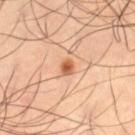Notes:
* location — the left thigh
* patient — male, in their mid- to late 60s
* imaging modality — 15 mm crop, total-body photography
* lesion diameter — ~2 mm (longest diameter)
* illumination — cross-polarized illumination